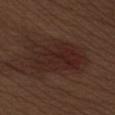Assessment: Imaged during a routine full-body skin examination; the lesion was not biopsied and no histopathology is available. Acquisition and patient details: The lesion is on the left upper arm. The subject is a male aged approximately 70. This image is a 15 mm lesion crop taken from a total-body photograph.